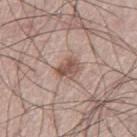The lesion was tiled from a total-body skin photograph and was not biopsied. Captured under white-light illumination. The lesion is on the right leg. A male patient aged 68–72. A 15 mm close-up tile from a total-body photography series done for melanoma screening.